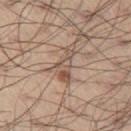Clinical impression: Imaged during a routine full-body skin examination; the lesion was not biopsied and no histopathology is available. Context: The lesion is on the leg. The total-body-photography lesion software estimated an average lesion color of about L≈54 a*≈16 b*≈26 (CIELAB), about 10 CIELAB-L* units darker than the surrounding skin, and a normalized border contrast of about 7. The analysis additionally found a border-irregularity index near 5.5/10, internal color variation of about 6 on a 0–10 scale, and peripheral color asymmetry of about 1. Imaged with white-light lighting. The patient is a male aged 53 to 57. The lesion's longest dimension is about 4.5 mm. Cropped from a whole-body photographic skin survey; the tile spans about 15 mm.